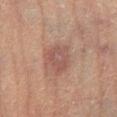notes = imaged on a skin check; not biopsied
lesion diameter = about 3.5 mm
lighting = cross-polarized
site = the left lower leg
subject = male, in their mid-80s or older
acquisition = ~15 mm crop, total-body skin-cancer survey